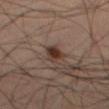{"site": "leg", "lesion_size": {"long_diameter_mm_approx": 2.5}, "lighting": "cross-polarized", "image": {"source": "total-body photography crop", "field_of_view_mm": 15}, "automated_metrics": {"area_mm2_approx": 4.5, "shape_asymmetry": 0.25, "color_variation_0_10": 5.0}, "patient": {"sex": "male", "age_approx": 65}}